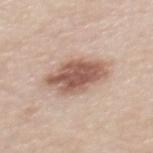| key | value |
|---|---|
| biopsy status | imaged on a skin check; not biopsied |
| image-analysis metrics | a within-lesion color-variation index near 5/10 |
| lighting | white-light |
| body site | the mid back |
| image | total-body-photography crop, ~15 mm field of view |
| patient | female, aged approximately 45 |
| diameter | ≈6 mm |